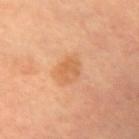Clinical impression: Recorded during total-body skin imaging; not selected for excision or biopsy. Context: Automated image analysis of the tile measured a footprint of about 5 mm² and two-axis asymmetry of about 0.15. It also reported a lesion color around L≈59 a*≈23 b*≈39 in CIELAB and a normalized border contrast of about 6. The analysis additionally found a border-irregularity rating of about 1.5/10, a within-lesion color-variation index near 2/10, and peripheral color asymmetry of about 1. The analysis additionally found a lesion-detection confidence of about 100/100. A close-up tile cropped from a whole-body skin photograph, about 15 mm across. A female subject aged around 70. The lesion is located on the right upper arm. Imaged with cross-polarized lighting. About 3 mm across.